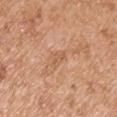Context:
The recorded lesion diameter is about 2.5 mm. A lesion tile, about 15 mm wide, cut from a 3D total-body photograph. The patient is a male about 55 years old. Located on the arm.